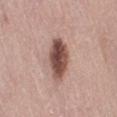About 5 mm across. The lesion is on the right thigh. This is a white-light tile. A lesion tile, about 15 mm wide, cut from a 3D total-body photograph. The patient is a female in their mid-60s. Automated tile analysis of the lesion measured an area of roughly 11 mm², a shape eccentricity near 0.85, and a symmetry-axis asymmetry near 0.15. The analysis additionally found an automated nevus-likeness rating near 95 out of 100 and a lesion-detection confidence of about 100/100.